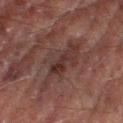<lesion>
  <biopsy_status>not biopsied; imaged during a skin examination</biopsy_status>
  <image>
    <source>total-body photography crop</source>
    <field_of_view_mm>15</field_of_view_mm>
  </image>
  <automated_metrics>
    <area_mm2_approx>14.0</area_mm2_approx>
    <eccentricity>0.9</eccentricity>
    <shape_asymmetry>0.45</shape_asymmetry>
  </automated_metrics>
  <patient>
    <sex>male</sex>
    <age_approx>70</age_approx>
  </patient>
  <site>leg</site>
  <lesion_size>
    <long_diameter_mm_approx>7.5</long_diameter_mm_approx>
  </lesion_size>
  <lighting>cross-polarized</lighting>
</lesion>